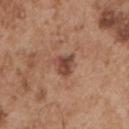Image and clinical context: Longest diameter approximately 3 mm. From the left upper arm. A male patient, about 55 years old. The lesion-visualizer software estimated a mean CIELAB color near L≈46 a*≈21 b*≈28, a lesion–skin lightness drop of about 11, and a lesion-to-skin contrast of about 8 (normalized; higher = more distinct). It also reported internal color variation of about 3.5 on a 0–10 scale and peripheral color asymmetry of about 1. The software also gave an automated nevus-likeness rating near 20 out of 100 and a lesion-detection confidence of about 100/100. A region of skin cropped from a whole-body photographic capture, roughly 15 mm wide. Captured under white-light illumination.